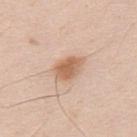Background:
The lesion is on the upper back. The patient is a male roughly 35 years of age. This image is a 15 mm lesion crop taken from a total-body photograph. An algorithmic analysis of the crop reported a footprint of about 7.5 mm² and a shape-asymmetry score of about 0.25 (0 = symmetric). The analysis additionally found a mean CIELAB color near L≈63 a*≈20 b*≈33, about 12 CIELAB-L* units darker than the surrounding skin, and a normalized lesion–skin contrast near 8. The analysis additionally found an automated nevus-likeness rating near 90 out of 100 and lesion-presence confidence of about 100/100.